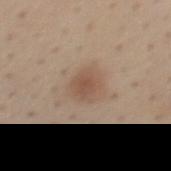patient: male, roughly 40 years of age | anatomic site: the back | image: ~15 mm tile from a whole-body skin photo.